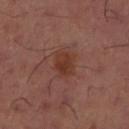Background: A lesion tile, about 15 mm wide, cut from a 3D total-body photograph. The lesion is located on the left thigh.